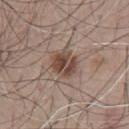| key | value |
|---|---|
| biopsy status | no biopsy performed (imaged during a skin exam) |
| tile lighting | white-light illumination |
| anatomic site | the chest |
| image | ~15 mm tile from a whole-body skin photo |
| size | ≈3.5 mm |
| automated metrics | an eccentricity of roughly 0.35 and a symmetry-axis asymmetry near 0.2; a detector confidence of about 100 out of 100 that the crop contains a lesion |
| patient | male, aged approximately 65 |